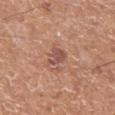The lesion was photographed on a routine skin check and not biopsied; there is no pathology result. A male patient, aged 53–57. The lesion is located on the left lower leg. Cropped from a total-body skin-imaging series; the visible field is about 15 mm.